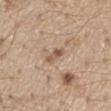Clinical impression:
Recorded during total-body skin imaging; not selected for excision or biopsy.
Context:
Located on the front of the torso. The subject is a male aged 68 to 72. Imaged with white-light lighting. A 15 mm close-up tile from a total-body photography series done for melanoma screening. Longest diameter approximately 3 mm.